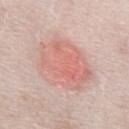Impression: Captured during whole-body skin photography for melanoma surveillance; the lesion was not biopsied. Clinical summary: The lesion is located on the front of the torso. A 15 mm crop from a total-body photograph taken for skin-cancer surveillance. About 7 mm across. A male subject aged 73 to 77.